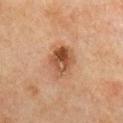notes=catalogued during a skin exam; not biopsied
image-analysis metrics=an average lesion color of about L≈41 a*≈19 b*≈29 (CIELAB), roughly 11 lightness units darker than nearby skin, and a normalized border contrast of about 8.5; an automated nevus-likeness rating near 50 out of 100 and a lesion-detection confidence of about 100/100
illumination=cross-polarized illumination
acquisition=~15 mm tile from a whole-body skin photo
site=the front of the torso
subject=female, roughly 60 years of age
lesion diameter=≈4 mm